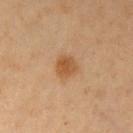- workup: catalogued during a skin exam; not biopsied
- image source: ~15 mm crop, total-body skin-cancer survey
- patient: female, about 50 years old
- anatomic site: the left upper arm